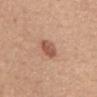<record>
<biopsy_status>not biopsied; imaged during a skin examination</biopsy_status>
<image>
  <source>total-body photography crop</source>
  <field_of_view_mm>15</field_of_view_mm>
</image>
<automated_metrics>
  <area_mm2_approx>5.0</area_mm2_approx>
  <eccentricity>0.55</eccentricity>
  <shape_asymmetry>0.2</shape_asymmetry>
  <border_irregularity_0_10>1.5</border_irregularity_0_10>
  <peripheral_color_asymmetry>1.5</peripheral_color_asymmetry>
  <lesion_detection_confidence_0_100>100</lesion_detection_confidence_0_100>
</automated_metrics>
<lesion_size>
  <long_diameter_mm_approx>2.5</long_diameter_mm_approx>
</lesion_size>
<patient>
  <sex>female</sex>
  <age_approx>55</age_approx>
</patient>
<lighting>white-light</lighting>
<site>chest</site>
</record>